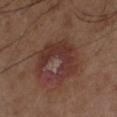Findings:
- follow-up — catalogued during a skin exam; not biopsied
- size — ~5.5 mm (longest diameter)
- patient — male, aged around 65
- body site — the leg
- lighting — cross-polarized illumination
- image source — ~15 mm tile from a whole-body skin photo